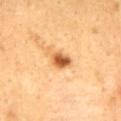<lesion>
  <biopsy_status>not biopsied; imaged during a skin examination</biopsy_status>
  <lighting>cross-polarized</lighting>
  <patient>
    <sex>male</sex>
    <age_approx>50</age_approx>
  </patient>
  <site>mid back</site>
  <image>
    <source>total-body photography crop</source>
    <field_of_view_mm>15</field_of_view_mm>
  </image>
</lesion>